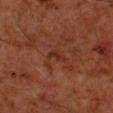This lesion was catalogued during total-body skin photography and was not selected for biopsy. The lesion is on the right forearm. Cropped from a whole-body photographic skin survey; the tile spans about 15 mm. The lesion's longest dimension is about 2.5 mm. A male subject, aged approximately 60.